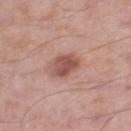Q: Was this lesion biopsied?
A: total-body-photography surveillance lesion; no biopsy
Q: Who is the patient?
A: male, about 55 years old
Q: What is the lesion's diameter?
A: ~3 mm (longest diameter)
Q: What is the anatomic site?
A: the left thigh
Q: What is the imaging modality?
A: ~15 mm crop, total-body skin-cancer survey